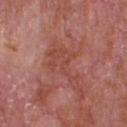Q: Was a biopsy performed?
A: catalogued during a skin exam; not biopsied